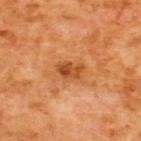| feature | finding |
|---|---|
| workup | no biopsy performed (imaged during a skin exam) |
| patient | male, in their mid- to late 60s |
| site | the upper back |
| image source | ~15 mm tile from a whole-body skin photo |
| tile lighting | cross-polarized illumination |